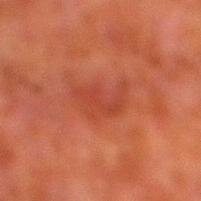{"biopsy_status": "not biopsied; imaged during a skin examination", "lesion_size": {"long_diameter_mm_approx": 5.0}, "lighting": "cross-polarized", "automated_metrics": {"area_mm2_approx": 9.0, "eccentricity": 0.85, "shape_asymmetry": 0.45, "cielab_L": 36, "cielab_a": 28, "cielab_b": 29, "vs_skin_contrast_norm": 5.0, "nevus_likeness_0_100": 0, "lesion_detection_confidence_0_100": 100}, "patient": {"sex": "male", "age_approx": 80}, "site": "leg", "image": {"source": "total-body photography crop", "field_of_view_mm": 15}}